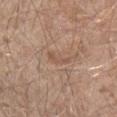- lesion size — ~3 mm (longest diameter)
- illumination — white-light
- subject — male, aged around 55
- location — the left forearm
- image — total-body-photography crop, ~15 mm field of view